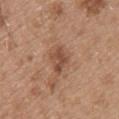Imaged during a routine full-body skin examination; the lesion was not biopsied and no histopathology is available. A 15 mm close-up extracted from a 3D total-body photography capture. The lesion is on the back. The patient is a male aged approximately 50. About 3 mm across. Imaged with white-light lighting. An algorithmic analysis of the crop reported an area of roughly 5 mm², an eccentricity of roughly 0.6, and a symmetry-axis asymmetry near 0.25. It also reported a lesion color around L≈48 a*≈21 b*≈30 in CIELAB and a lesion-to-skin contrast of about 7.5 (normalized; higher = more distinct). And it measured border irregularity of about 2.5 on a 0–10 scale, internal color variation of about 3 on a 0–10 scale, and radial color variation of about 1. The analysis additionally found a classifier nevus-likeness of about 20/100 and lesion-presence confidence of about 100/100.